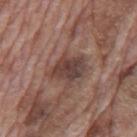workup=imaged on a skin check; not biopsied
site=the mid back
image source=~15 mm crop, total-body skin-cancer survey
patient=male, in their 70s
diameter=≈4 mm
lighting=white-light illumination